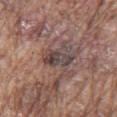follow-up=no biopsy performed (imaged during a skin exam) | site=the chest | size=≈3.5 mm | subject=male, aged 73 to 77 | acquisition=15 mm crop, total-body photography | automated lesion analysis=a lesion area of about 6 mm², an eccentricity of roughly 0.85, and two-axis asymmetry of about 0.35; an average lesion color of about L≈41 a*≈14 b*≈17 (CIELAB), a lesion–skin lightness drop of about 11, and a normalized border contrast of about 9; border irregularity of about 4 on a 0–10 scale, a color-variation rating of about 7/10, and radial color variation of about 2.5 | lighting=white-light.